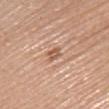This lesion was catalogued during total-body skin photography and was not selected for biopsy.
The patient is a female in their mid- to late 60s.
Cropped from a total-body skin-imaging series; the visible field is about 15 mm.
The lesion is located on the back.